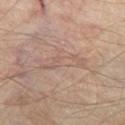biopsy status = imaged on a skin check; not biopsied
diameter = ~6 mm (longest diameter)
subject = in their mid- to late 50s
body site = the left thigh
illumination = cross-polarized
image = 15 mm crop, total-body photography